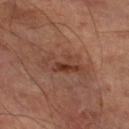Case summary:
- follow-up: imaged on a skin check; not biopsied
- image: ~15 mm tile from a whole-body skin photo
- tile lighting: cross-polarized illumination
- lesion diameter: about 5.5 mm
- location: the right lower leg
- subject: male, aged around 70